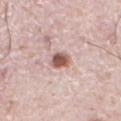follow-up — imaged on a skin check; not biopsied
tile lighting — white-light
body site — the abdomen
patient — male, roughly 75 years of age
image source — 15 mm crop, total-body photography
lesion diameter — ~2.5 mm (longest diameter)
automated metrics — a footprint of about 4.5 mm² and an eccentricity of roughly 0.6; a lesion color around L≈57 a*≈21 b*≈24 in CIELAB and a lesion–skin lightness drop of about 17; a classifier nevus-likeness of about 95/100